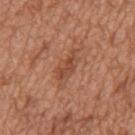Clinical impression:
The lesion was photographed on a routine skin check and not biopsied; there is no pathology result.
Acquisition and patient details:
The lesion is located on the mid back. An algorithmic analysis of the crop reported an eccentricity of roughly 0.9 and a symmetry-axis asymmetry near 0.35. This image is a 15 mm lesion crop taken from a total-body photograph. A male patient roughly 65 years of age.